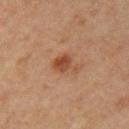Recorded during total-body skin imaging; not selected for excision or biopsy. A 15 mm close-up tile from a total-body photography series done for melanoma screening. Captured under cross-polarized illumination. On the mid back. The subject is a male approximately 65 years of age. An algorithmic analysis of the crop reported an outline eccentricity of about 0.75 (0 = round, 1 = elongated). The software also gave a within-lesion color-variation index near 4.5/10 and radial color variation of about 1.5.